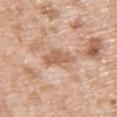Recorded during total-body skin imaging; not selected for excision or biopsy. The patient is a male aged around 50. The lesion is located on the chest. A region of skin cropped from a whole-body photographic capture, roughly 15 mm wide.